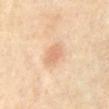Imaged during a routine full-body skin examination; the lesion was not biopsied and no histopathology is available. The patient is a male in their 70s. A 15 mm crop from a total-body photograph taken for skin-cancer surveillance. The lesion is located on the abdomen.